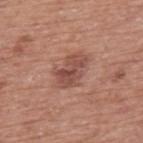workup — no biopsy performed (imaged during a skin exam)
diameter — ~4.5 mm (longest diameter)
subject — male, about 75 years old
illumination — white-light
anatomic site — the upper back
imaging modality — total-body-photography crop, ~15 mm field of view